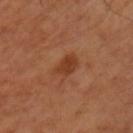Assessment:
Imaged during a routine full-body skin examination; the lesion was not biopsied and no histopathology is available.
Image and clinical context:
From the left upper arm. A lesion tile, about 15 mm wide, cut from a 3D total-body photograph. A male subject, in their 50s.